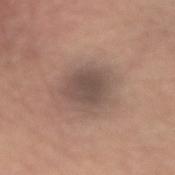Assessment: Part of a total-body skin-imaging series; this lesion was reviewed on a skin check and was not flagged for biopsy. Clinical summary: About 4 mm across. Automated image analysis of the tile measured an outline eccentricity of about 0.55 (0 = round, 1 = elongated). The software also gave a lesion–skin lightness drop of about 10 and a lesion-to-skin contrast of about 7.5 (normalized; higher = more distinct). The analysis additionally found a border-irregularity index near 2/10, a color-variation rating of about 2.5/10, and a peripheral color-asymmetry measure near 1. And it measured a nevus-likeness score of about 20/100 and a detector confidence of about 100 out of 100 that the crop contains a lesion. Imaged with white-light lighting. Located on the right upper arm. A region of skin cropped from a whole-body photographic capture, roughly 15 mm wide. The subject is a male aged 68 to 72.